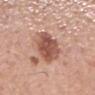No biopsy was performed on this lesion — it was imaged during a full skin examination and was not determined to be concerning. On the mid back. This image is a 15 mm lesion crop taken from a total-body photograph. The subject is a male roughly 60 years of age.